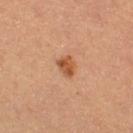<tbp_lesion>
  <site>right thigh</site>
  <lighting>cross-polarized</lighting>
  <image>
    <source>total-body photography crop</source>
    <field_of_view_mm>15</field_of_view_mm>
  </image>
  <lesion_size>
    <long_diameter_mm_approx>2.5</long_diameter_mm_approx>
  </lesion_size>
  <automated_metrics>
    <area_mm2_approx>4.0</area_mm2_approx>
    <eccentricity>0.65</eccentricity>
    <shape_asymmetry>0.3</shape_asymmetry>
    <cielab_L>47</cielab_L>
    <cielab_a>23</cielab_a>
    <cielab_b>35</cielab_b>
    <vs_skin_contrast_norm>8.5</vs_skin_contrast_norm>
    <border_irregularity_0_10>2.5</border_irregularity_0_10>
    <color_variation_0_10>3.0</color_variation_0_10>
    <peripheral_color_asymmetry>1.0</peripheral_color_asymmetry>
    <nevus_likeness_0_100>80</nevus_likeness_0_100>
  </automated_metrics>
  <patient>
    <sex>female</sex>
    <age_approx>30</age_approx>
  </patient>
</tbp_lesion>